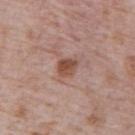The recorded lesion diameter is about 2.5 mm.
Imaged with white-light lighting.
A 15 mm close-up tile from a total-body photography series done for melanoma screening.
The patient is a male about 70 years old.
From the abdomen.